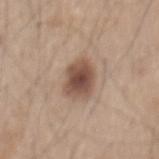TBP lesion metrics: a mean CIELAB color near L≈50 a*≈17 b*≈26, a lesion–skin lightness drop of about 14, and a lesion-to-skin contrast of about 10 (normalized; higher = more distinct) | size: ~4.5 mm (longest diameter) | image source: ~15 mm crop, total-body skin-cancer survey | illumination: white-light | site: the back | patient: male, aged approximately 45.